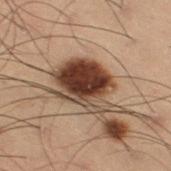imaging modality = ~15 mm tile from a whole-body skin photo
illumination = cross-polarized
image-analysis metrics = a lesion–skin lightness drop of about 16; a nevus-likeness score of about 100/100 and a detector confidence of about 100 out of 100 that the crop contains a lesion
lesion size = ≈7.5 mm
patient = male, aged approximately 55
site = the right thigh